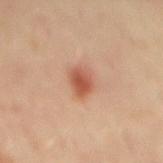This lesion was catalogued during total-body skin photography and was not selected for biopsy. Approximately 3 mm at its widest. A 15 mm close-up extracted from a 3D total-body photography capture. A male subject aged around 50. Located on the mid back. Captured under cross-polarized illumination. The total-body-photography lesion software estimated an area of roughly 6 mm², an outline eccentricity of about 0.7 (0 = round, 1 = elongated), and a shape-asymmetry score of about 0.2 (0 = symmetric). The analysis additionally found about 12 CIELAB-L* units darker than the surrounding skin and a lesion-to-skin contrast of about 8 (normalized; higher = more distinct).